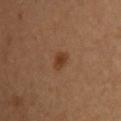Assessment: Imaged during a routine full-body skin examination; the lesion was not biopsied and no histopathology is available. Acquisition and patient details: The lesion-visualizer software estimated a border-irregularity index near 1.5/10. And it measured a nevus-likeness score of about 90/100. A female patient roughly 30 years of age. The lesion is on the chest. Captured under cross-polarized illumination. Cropped from a whole-body photographic skin survey; the tile spans about 15 mm. The lesion's longest dimension is about 2.5 mm.